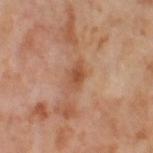This lesion was catalogued during total-body skin photography and was not selected for biopsy. This image is a 15 mm lesion crop taken from a total-body photograph. A female patient approximately 55 years of age. Captured under cross-polarized illumination. The lesion is located on the right thigh.